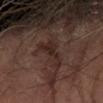Clinical impression: The lesion was tiled from a total-body skin photograph and was not biopsied. Clinical summary: The lesion-visualizer software estimated a footprint of about 7 mm², a shape eccentricity near 0.9, and two-axis asymmetry of about 0.55. The software also gave a lesion color around L≈24 a*≈16 b*≈18 in CIELAB, a lesion–skin lightness drop of about 7, and a normalized lesion–skin contrast near 8. It also reported a detector confidence of about 90 out of 100 that the crop contains a lesion. The subject is a male roughly 65 years of age. Imaged with cross-polarized lighting. A roughly 15 mm field-of-view crop from a total-body skin photograph. From the left forearm.